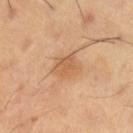follow-up = no biopsy performed (imaged during a skin exam) | site = the right thigh | imaging modality = total-body-photography crop, ~15 mm field of view | patient = male, aged 53 to 57 | TBP lesion metrics = a mean CIELAB color near L≈57 a*≈20 b*≈36, about 8 CIELAB-L* units darker than the surrounding skin, and a normalized lesion–skin contrast near 5.5; a detector confidence of about 100 out of 100 that the crop contains a lesion | tile lighting = cross-polarized illumination.